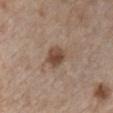Context:
A female subject about 40 years old. A 15 mm close-up tile from a total-body photography series done for melanoma screening. Measured at roughly 3 mm in maximum diameter. The lesion is on the chest. The tile uses white-light illumination.